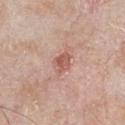Recorded during total-body skin imaging; not selected for excision or biopsy.
An algorithmic analysis of the crop reported an outline eccentricity of about 0.6 (0 = round, 1 = elongated) and a symmetry-axis asymmetry near 0.35. It also reported a mean CIELAB color near L≈56 a*≈24 b*≈26, about 11 CIELAB-L* units darker than the surrounding skin, and a lesion-to-skin contrast of about 7 (normalized; higher = more distinct).
Located on the chest.
This is a white-light tile.
The patient is a male roughly 65 years of age.
A 15 mm crop from a total-body photograph taken for skin-cancer surveillance.
The recorded lesion diameter is about 2.5 mm.